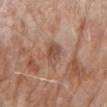No biopsy was performed on this lesion — it was imaged during a full skin examination and was not determined to be concerning.
A female patient aged around 85 or older.
An algorithmic analysis of the crop reported a nevus-likeness score of about 0/100.
The lesion is on the right upper arm.
This is a white-light tile.
Cropped from a whole-body photographic skin survey; the tile spans about 15 mm.
The recorded lesion diameter is about 3 mm.